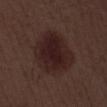Q: Was a biopsy performed?
A: imaged on a skin check; not biopsied
Q: Automated lesion metrics?
A: an outline eccentricity of about 0.7 (0 = round, 1 = elongated); roughly 8 lightness units darker than nearby skin and a lesion-to-skin contrast of about 9.5 (normalized; higher = more distinct)
Q: What lighting was used for the tile?
A: white-light illumination
Q: Who is the patient?
A: male, aged 68–72
Q: How was this image acquired?
A: ~15 mm crop, total-body skin-cancer survey
Q: How large is the lesion?
A: about 7 mm
Q: Lesion location?
A: the leg